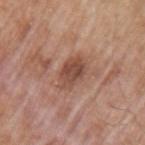Impression: The lesion was photographed on a routine skin check and not biopsied; there is no pathology result. Background: Captured under white-light illumination. Located on the left upper arm. A male subject, aged 63 to 67. A lesion tile, about 15 mm wide, cut from a 3D total-body photograph. The lesion's longest dimension is about 4 mm.